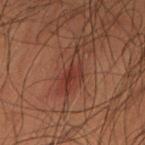{
  "biopsy_status": "not biopsied; imaged during a skin examination",
  "image": {
    "source": "total-body photography crop",
    "field_of_view_mm": 15
  },
  "patient": {
    "sex": "male",
    "age_approx": 50
  },
  "lighting": "cross-polarized",
  "lesion_size": {
    "long_diameter_mm_approx": 3.5
  },
  "site": "left thigh"
}